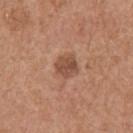| field | value |
|---|---|
| workup | no biopsy performed (imaged during a skin exam) |
| size | ~2.5 mm (longest diameter) |
| location | the right upper arm |
| acquisition | 15 mm crop, total-body photography |
| patient | male, aged 73–77 |